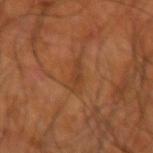| feature | finding |
|---|---|
| follow-up | imaged on a skin check; not biopsied |
| site | the right upper arm |
| size | ≈3 mm |
| patient | male, in their mid-60s |
| acquisition | ~15 mm tile from a whole-body skin photo |
| lighting | cross-polarized |
| automated metrics | an average lesion color of about L≈40 a*≈23 b*≈35 (CIELAB), roughly 6 lightness units darker than nearby skin, and a normalized lesion–skin contrast near 5.5; a border-irregularity rating of about 3.5/10, internal color variation of about 2 on a 0–10 scale, and radial color variation of about 0.5 |